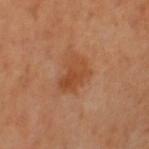<tbp_lesion>
  <biopsy_status>not biopsied; imaged during a skin examination</biopsy_status>
  <image>
    <source>total-body photography crop</source>
    <field_of_view_mm>15</field_of_view_mm>
  </image>
  <patient>
    <sex>female</sex>
    <age_approx>55</age_approx>
  </patient>
  <lighting>cross-polarized</lighting>
  <site>left thigh</site>
  <lesion_size>
    <long_diameter_mm_approx>4.0</long_diameter_mm_approx>
  </lesion_size>
</tbp_lesion>